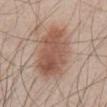follow-up=total-body-photography surveillance lesion; no biopsy